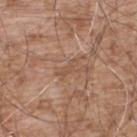Impression:
Imaged during a routine full-body skin examination; the lesion was not biopsied and no histopathology is available.
Context:
This is a white-light tile. The subject is a male roughly 55 years of age. This image is a 15 mm lesion crop taken from a total-body photograph. The lesion is located on the back. Measured at roughly 3 mm in maximum diameter.